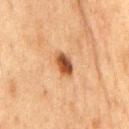{
  "biopsy_status": "not biopsied; imaged during a skin examination",
  "lighting": "cross-polarized",
  "site": "chest",
  "patient": {
    "sex": "male",
    "age_approx": 75
  },
  "lesion_size": {
    "long_diameter_mm_approx": 3.0
  },
  "image": {
    "source": "total-body photography crop",
    "field_of_view_mm": 15
  }
}